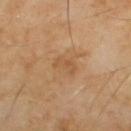This lesion was catalogued during total-body skin photography and was not selected for biopsy.
The patient is a male aged around 55.
Cropped from a whole-body photographic skin survey; the tile spans about 15 mm.
This is a cross-polarized tile.
Automated image analysis of the tile measured a lesion color around L≈49 a*≈19 b*≈34 in CIELAB, a lesion–skin lightness drop of about 6, and a normalized border contrast of about 5. The analysis additionally found peripheral color asymmetry of about 0. The analysis additionally found an automated nevus-likeness rating near 0 out of 100 and lesion-presence confidence of about 100/100.
The lesion is located on the upper back.